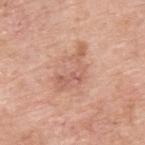  biopsy_status: not biopsied; imaged during a skin examination